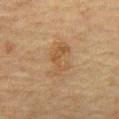Part of a total-body skin-imaging series; this lesion was reviewed on a skin check and was not flagged for biopsy.
The subject is a male roughly 75 years of age.
A close-up tile cropped from a whole-body skin photograph, about 15 mm across.
Measured at roughly 5.5 mm in maximum diameter.
The lesion is on the abdomen.